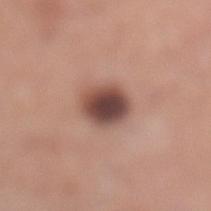Q: Who is the patient?
A: female, roughly 30 years of age
Q: What is the anatomic site?
A: the left lower leg
Q: What lighting was used for the tile?
A: white-light illumination
Q: Lesion size?
A: ~4 mm (longest diameter)
Q: How was this image acquired?
A: ~15 mm tile from a whole-body skin photo